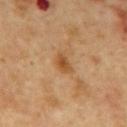Imaged during a routine full-body skin examination; the lesion was not biopsied and no histopathology is available.
The patient is a male aged approximately 50.
A 15 mm close-up extracted from a 3D total-body photography capture.
Captured under cross-polarized illumination.
From the mid back.